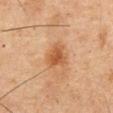biopsy status: imaged on a skin check; not biopsied
automated metrics: an outline eccentricity of about 0.4 (0 = round, 1 = elongated) and a shape-asymmetry score of about 0.3 (0 = symmetric); a color-variation rating of about 2.5/10 and radial color variation of about 0.5; a classifier nevus-likeness of about 70/100 and a lesion-detection confidence of about 100/100
anatomic site: the abdomen
subject: male, in their mid-70s
illumination: cross-polarized illumination
lesion diameter: about 3 mm
image: total-body-photography crop, ~15 mm field of view